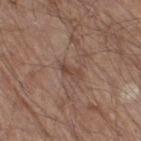Q: Is there a histopathology result?
A: catalogued during a skin exam; not biopsied